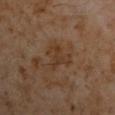Notes:
– notes · no biopsy performed (imaged during a skin exam)
– image source · 15 mm crop, total-body photography
– subject · male, aged 58 to 62
– location · the left upper arm